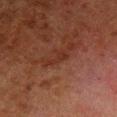Findings:
- workup — catalogued during a skin exam; not biopsied
- illumination — cross-polarized illumination
- image — 15 mm crop, total-body photography
- anatomic site — the left lower leg
- subject — male, in their 80s